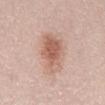workup = imaged on a skin check; not biopsied
patient = female, aged 48 to 52
lesion diameter = ≈6 mm
lighting = white-light
site = the abdomen
image-analysis metrics = two-axis asymmetry of about 0.25; an average lesion color of about L≈61 a*≈21 b*≈28 (CIELAB) and a normalized lesion–skin contrast near 7.5; a classifier nevus-likeness of about 90/100 and lesion-presence confidence of about 100/100
imaging modality = total-body-photography crop, ~15 mm field of view